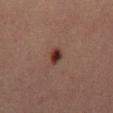  biopsy_status: not biopsied; imaged during a skin examination
  lighting: cross-polarized
  patient:
    sex: male
    age_approx: 30
  site: mid back
  automated_metrics:
    area_mm2_approx: 3.0
    shape_asymmetry: 0.2
    border_irregularity_0_10: 1.5
    color_variation_0_10: 3.5
  image:
    source: total-body photography crop
    field_of_view_mm: 15